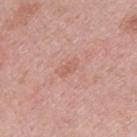Recorded during total-body skin imaging; not selected for excision or biopsy.
The lesion is located on the upper back.
The lesion-visualizer software estimated an area of roughly 3 mm² and two-axis asymmetry of about 0.35. And it measured an automated nevus-likeness rating near 0 out of 100 and lesion-presence confidence of about 100/100.
A close-up tile cropped from a whole-body skin photograph, about 15 mm across.
A male subject, in their mid-20s.
Captured under white-light illumination.
About 3 mm across.